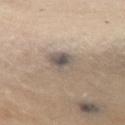workup — catalogued during a skin exam; not biopsied
diameter — ~3.5 mm (longest diameter)
body site — the chest
automated metrics — an area of roughly 6.5 mm²; a lesion color around L≈54 a*≈9 b*≈20 in CIELAB; a within-lesion color-variation index near 7/10 and radial color variation of about 2; a lesion-detection confidence of about 55/100
image source — ~15 mm tile from a whole-body skin photo
patient — female, about 70 years old